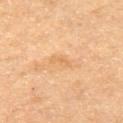| key | value |
|---|---|
| biopsy status | total-body-photography surveillance lesion; no biopsy |
| subject | female, aged 53–57 |
| location | the leg |
| illumination | cross-polarized illumination |
| image | 15 mm crop, total-body photography |